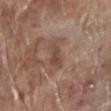workup: no biopsy performed (imaged during a skin exam)
patient: male, about 70 years old
image source: total-body-photography crop, ~15 mm field of view
location: the left lower leg
lesion size: ~4 mm (longest diameter)
illumination: white-light illumination
automated metrics: an outline eccentricity of about 0.9 (0 = round, 1 = elongated) and two-axis asymmetry of about 0.35; a mean CIELAB color near L≈46 a*≈18 b*≈24, roughly 8 lightness units darker than nearby skin, and a lesion-to-skin contrast of about 6.5 (normalized; higher = more distinct); a nevus-likeness score of about 0/100 and a detector confidence of about 95 out of 100 that the crop contains a lesion